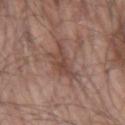The lesion was photographed on a routine skin check and not biopsied; there is no pathology result.
Located on the right upper arm.
A male subject, aged approximately 65.
The tile uses white-light illumination.
Automated image analysis of the tile measured a shape eccentricity near 0.7 and a symmetry-axis asymmetry near 0.5. It also reported an average lesion color of about L≈45 a*≈19 b*≈24 (CIELAB) and a normalized lesion–skin contrast near 6.5. The analysis additionally found a border-irregularity index near 6.5/10 and a peripheral color-asymmetry measure near 1.
The lesion's longest dimension is about 4 mm.
A 15 mm crop from a total-body photograph taken for skin-cancer surveillance.